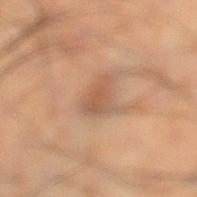Recorded during total-body skin imaging; not selected for excision or biopsy.
Automated image analysis of the tile measured a mean CIELAB color near L≈54 a*≈20 b*≈32, roughly 9 lightness units darker than nearby skin, and a lesion-to-skin contrast of about 6 (normalized; higher = more distinct).
From the left lower leg.
A male patient, approximately 40 years of age.
Measured at roughly 3.5 mm in maximum diameter.
A 15 mm close-up tile from a total-body photography series done for melanoma screening.